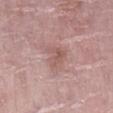Notes:
- workup · total-body-photography surveillance lesion; no biopsy
- site · the right lower leg
- image source · total-body-photography crop, ~15 mm field of view
- illumination · white-light illumination
- subject · female, aged approximately 70
- lesion size · ≈3 mm